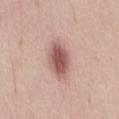The lesion was tiled from a total-body skin photograph and was not biopsied.
Automated tile analysis of the lesion measured a shape eccentricity near 0.8 and two-axis asymmetry of about 0.1. The software also gave an average lesion color of about L≈57 a*≈23 b*≈22 (CIELAB) and about 14 CIELAB-L* units darker than the surrounding skin. The software also gave border irregularity of about 1.5 on a 0–10 scale and peripheral color asymmetry of about 1.
A 15 mm crop from a total-body photograph taken for skin-cancer surveillance.
A female patient, aged approximately 65.
On the abdomen.
Imaged with white-light lighting.
Measured at roughly 5 mm in maximum diameter.